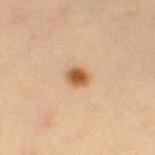follow-up = imaged on a skin check; not biopsied
diameter = ~2.5 mm (longest diameter)
location = the left thigh
imaging modality = ~15 mm crop, total-body skin-cancer survey
lighting = cross-polarized
subject = female, roughly 40 years of age